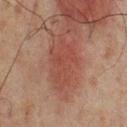Assessment: This lesion was catalogued during total-body skin photography and was not selected for biopsy. Context: A 15 mm close-up tile from a total-body photography series done for melanoma screening. About 6 mm across. Captured under cross-polarized illumination. The lesion is located on the back. A male subject aged 63–67.